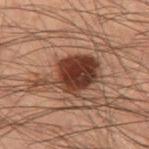biopsy_status: not biopsied; imaged during a skin examination
site: leg
patient:
  sex: male
  age_approx: 55
lesion_size:
  long_diameter_mm_approx: 6.5
lighting: cross-polarized
image:
  source: total-body photography crop
  field_of_view_mm: 15